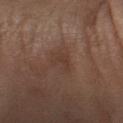Impression: Recorded during total-body skin imaging; not selected for excision or biopsy. Acquisition and patient details: A male patient, in their 60s. A close-up tile cropped from a whole-body skin photograph, about 15 mm across. Approximately 3 mm at its widest. Automated image analysis of the tile measured internal color variation of about 2 on a 0–10 scale and peripheral color asymmetry of about 1. The lesion is on the left forearm.